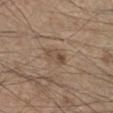Assessment: No biopsy was performed on this lesion — it was imaged during a full skin examination and was not determined to be concerning. Background: A 15 mm close-up tile from a total-body photography series done for melanoma screening. The lesion's longest dimension is about 3 mm. The tile uses white-light illumination. From the right lower leg. A male subject roughly 45 years of age. The total-body-photography lesion software estimated a lesion color around L≈48 a*≈15 b*≈27 in CIELAB, a lesion–skin lightness drop of about 8, and a normalized lesion–skin contrast near 6. And it measured a border-irregularity index near 2.5/10 and peripheral color asymmetry of about 2.